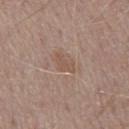<lesion>
  <biopsy_status>not biopsied; imaged during a skin examination</biopsy_status>
  <lighting>white-light</lighting>
  <lesion_size>
    <long_diameter_mm_approx>3.0</long_diameter_mm_approx>
  </lesion_size>
  <site>chest</site>
  <patient>
    <sex>male</sex>
    <age_approx>75</age_approx>
  </patient>
  <image>
    <source>total-body photography crop</source>
    <field_of_view_mm>15</field_of_view_mm>
  </image>
</lesion>